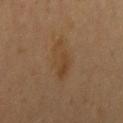biopsy status: imaged on a skin check; not biopsied | patient: male, about 40 years old | tile lighting: cross-polarized | acquisition: 15 mm crop, total-body photography | anatomic site: the right upper arm.